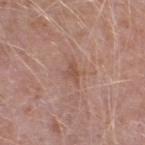<lesion>
  <biopsy_status>not biopsied; imaged during a skin examination</biopsy_status>
</lesion>